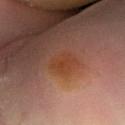| feature | finding |
|---|---|
| follow-up | no biopsy performed (imaged during a skin exam) |
| subject | male, in their mid- to late 60s |
| image | total-body-photography crop, ~15 mm field of view |
| lesion diameter | ~3 mm (longest diameter) |